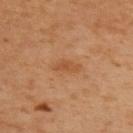biopsy status = catalogued during a skin exam; not biopsied
subject = male, roughly 60 years of age
body site = the upper back
image = ~15 mm crop, total-body skin-cancer survey